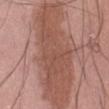| key | value |
|---|---|
| workup | catalogued during a skin exam; not biopsied |
| lighting | white-light |
| anatomic site | the front of the torso |
| lesion diameter | ~14.5 mm (longest diameter) |
| imaging modality | total-body-photography crop, ~15 mm field of view |
| subject | male, aged 48–52 |